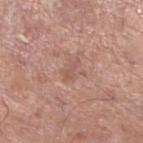Notes:
* biopsy status — catalogued during a skin exam; not biopsied
* image — ~15 mm crop, total-body skin-cancer survey
* illumination — white-light
* size — about 2.5 mm
* subject — male, aged around 65
* image-analysis metrics — a footprint of about 2.5 mm², an eccentricity of roughly 0.9, and a symmetry-axis asymmetry near 0.6; an average lesion color of about L≈55 a*≈21 b*≈26 (CIELAB) and roughly 6 lightness units darker than nearby skin; a nevus-likeness score of about 0/100
* site — the leg